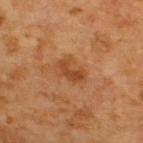Clinical impression: Imaged during a routine full-body skin examination; the lesion was not biopsied and no histopathology is available. Background: Captured under cross-polarized illumination. Located on the upper back. A 15 mm close-up extracted from a 3D total-body photography capture. The lesion's longest dimension is about 3.5 mm. A male patient aged approximately 65.